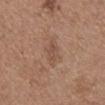Captured during whole-body skin photography for melanoma surveillance; the lesion was not biopsied.